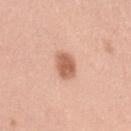The lesion was photographed on a routine skin check and not biopsied; there is no pathology result.
Automated tile analysis of the lesion measured a footprint of about 6.5 mm², an outline eccentricity of about 0.55 (0 = round, 1 = elongated), and a symmetry-axis asymmetry near 0.2. It also reported an average lesion color of about L≈61 a*≈24 b*≈32 (CIELAB) and a normalized lesion–skin contrast near 8.5.
Cropped from a total-body skin-imaging series; the visible field is about 15 mm.
The tile uses white-light illumination.
Longest diameter approximately 3 mm.
The lesion is located on the left upper arm.
A female patient, in their mid- to late 30s.